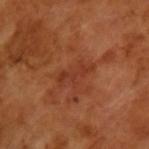Q: Was this lesion biopsied?
A: total-body-photography surveillance lesion; no biopsy
Q: What is the lesion's diameter?
A: ~4 mm (longest diameter)
Q: Patient demographics?
A: male, approximately 65 years of age
Q: What lighting was used for the tile?
A: cross-polarized
Q: How was this image acquired?
A: ~15 mm tile from a whole-body skin photo